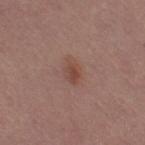biopsy status: imaged on a skin check; not biopsied | tile lighting: white-light illumination | acquisition: total-body-photography crop, ~15 mm field of view | body site: the right thigh | patient: female, aged approximately 50.